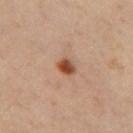Clinical impression: The lesion was photographed on a routine skin check and not biopsied; there is no pathology result.